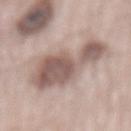| feature | finding |
|---|---|
| biopsy status | total-body-photography surveillance lesion; no biopsy |
| lighting | white-light illumination |
| imaging modality | total-body-photography crop, ~15 mm field of view |
| site | the mid back |
| size | about 8 mm |
| patient | male, aged around 65 |